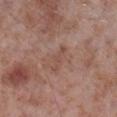Assessment: The lesion was photographed on a routine skin check and not biopsied; there is no pathology result. Background: This image is a 15 mm lesion crop taken from a total-body photograph. The tile uses white-light illumination. The recorded lesion diameter is about 3.5 mm. The lesion is on the right lower leg. The patient is a male aged approximately 55.